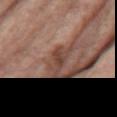| key | value |
|---|---|
| biopsy status | catalogued during a skin exam; not biopsied |
| subject | female, approximately 70 years of age |
| lighting | white-light |
| image-analysis metrics | a classifier nevus-likeness of about 0/100 and a lesion-detection confidence of about 90/100 |
| site | the chest |
| imaging modality | ~15 mm crop, total-body skin-cancer survey |